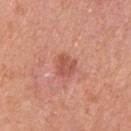Imaged during a routine full-body skin examination; the lesion was not biopsied and no histopathology is available. On the left upper arm. The subject is a male in their mid- to late 50s. A close-up tile cropped from a whole-body skin photograph, about 15 mm across.